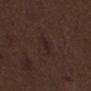workup = imaged on a skin check; not biopsied | location = the mid back | subject = male, about 70 years old | image = ~15 mm crop, total-body skin-cancer survey.